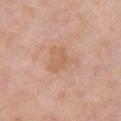- workup · catalogued during a skin exam; not biopsied
- lighting · white-light illumination
- patient · female, in their 60s
- site · the chest
- image source · 15 mm crop, total-body photography